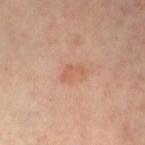Impression:
Recorded during total-body skin imaging; not selected for excision or biopsy.
Image and clinical context:
The lesion is located on the left thigh. A female patient, aged 48 to 52. About 3 mm across. A region of skin cropped from a whole-body photographic capture, roughly 15 mm wide.